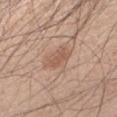Imaged during a routine full-body skin examination; the lesion was not biopsied and no histopathology is available.
A male subject, in their 30s.
On the left upper arm.
A roughly 15 mm field-of-view crop from a total-body skin photograph.
The tile uses white-light illumination.
Approximately 3.5 mm at its widest.